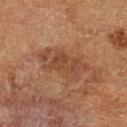Captured during whole-body skin photography for melanoma surveillance; the lesion was not biopsied.
The lesion is on the left lower leg.
A roughly 15 mm field-of-view crop from a total-body skin photograph.
A male subject, aged 73–77.